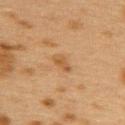Clinical impression: Imaged during a routine full-body skin examination; the lesion was not biopsied and no histopathology is available. Background: A lesion tile, about 15 mm wide, cut from a 3D total-body photograph. From the upper back. The tile uses cross-polarized illumination. A female subject approximately 40 years of age. About 3 mm across.